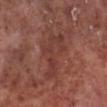Notes:
* notes · no biopsy performed (imaged during a skin exam)
* tile lighting · white-light illumination
* location · the left lower leg
* subject · male, aged 53–57
* imaging modality · ~15 mm tile from a whole-body skin photo
* lesion diameter · about 6.5 mm
* automated metrics · a lesion area of about 14 mm², a shape eccentricity near 0.85, and a shape-asymmetry score of about 0.55 (0 = symmetric); a border-irregularity rating of about 7/10, internal color variation of about 4.5 on a 0–10 scale, and radial color variation of about 1.5; an automated nevus-likeness rating near 0 out of 100 and a lesion-detection confidence of about 95/100